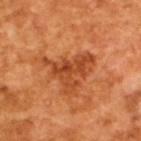  biopsy_status: not biopsied; imaged during a skin examination
  automated_metrics:
    vs_skin_darker_L: 10.0
    vs_skin_contrast_norm: 7.5
    border_irregularity_0_10: 7.5
    color_variation_0_10: 4.5
  patient:
    sex: male
    age_approx: 65
  lighting: cross-polarized
  image:
    source: total-body photography crop
    field_of_view_mm: 15